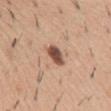Clinical impression:
The lesion was photographed on a routine skin check and not biopsied; there is no pathology result.
Acquisition and patient details:
On the abdomen. Cropped from a total-body skin-imaging series; the visible field is about 15 mm. The patient is a male roughly 40 years of age.